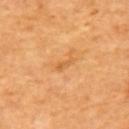Captured during whole-body skin photography for melanoma surveillance; the lesion was not biopsied. Located on the upper back. The subject is a male aged 63–67. A lesion tile, about 15 mm wide, cut from a 3D total-body photograph.